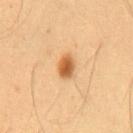workup: imaged on a skin check; not biopsied | patient: male, in their mid-50s | illumination: cross-polarized | image-analysis metrics: an area of roughly 5 mm² and an outline eccentricity of about 0.6 (0 = round, 1 = elongated); roughly 15 lightness units darker than nearby skin and a lesion-to-skin contrast of about 9.5 (normalized; higher = more distinct); an automated nevus-likeness rating near 100 out of 100 and lesion-presence confidence of about 100/100 | anatomic site: the chest | image: ~15 mm crop, total-body skin-cancer survey.